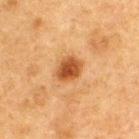Findings:
– notes — catalogued during a skin exam; not biopsied
– subject — male, aged approximately 75
– location — the back
– illumination — cross-polarized illumination
– diameter — about 3 mm
– image — ~15 mm tile from a whole-body skin photo